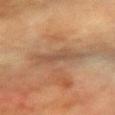Assessment:
The lesion was tiled from a total-body skin photograph and was not biopsied.
Background:
The lesion is on the right forearm. Automated image analysis of the tile measured an area of roughly 7 mm², a shape eccentricity near 0.85, and a shape-asymmetry score of about 0.25 (0 = symmetric). The software also gave a lesion color around L≈45 a*≈16 b*≈28 in CIELAB, a lesion–skin lightness drop of about 6, and a normalized border contrast of about 5.5. The software also gave border irregularity of about 3.5 on a 0–10 scale. Imaged with cross-polarized lighting. Measured at roughly 4 mm in maximum diameter. This image is a 15 mm lesion crop taken from a total-body photograph. A female subject, aged 53 to 57.